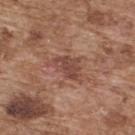notes: total-body-photography surveillance lesion; no biopsy | tile lighting: white-light | imaging modality: ~15 mm tile from a whole-body skin photo | anatomic site: the back | lesion size: ≈4 mm | TBP lesion metrics: a mean CIELAB color near L≈46 a*≈22 b*≈26, roughly 9 lightness units darker than nearby skin, and a normalized lesion–skin contrast near 7; a classifier nevus-likeness of about 0/100 and lesion-presence confidence of about 100/100 | patient: male, in their mid-70s.